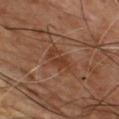Imaged during a routine full-body skin examination; the lesion was not biopsied and no histopathology is available. The lesion is on the chest. Cropped from a total-body skin-imaging series; the visible field is about 15 mm. Longest diameter approximately 3.5 mm. The patient is a male about 55 years old. Automated tile analysis of the lesion measured a lesion area of about 7 mm² and two-axis asymmetry of about 0.35. The analysis additionally found an average lesion color of about L≈35 a*≈20 b*≈29 (CIELAB) and a lesion–skin lightness drop of about 6. And it measured an automated nevus-likeness rating near 0 out of 100 and lesion-presence confidence of about 100/100. Captured under cross-polarized illumination.